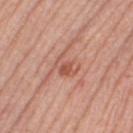{"biopsy_status": "not biopsied; imaged during a skin examination", "site": "right thigh", "image": {"source": "total-body photography crop", "field_of_view_mm": 15}, "lighting": "white-light", "patient": {"sex": "female", "age_approx": 65}}